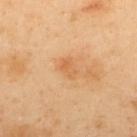The lesion was tiled from a total-body skin photograph and was not biopsied. Measured at roughly 2.5 mm in maximum diameter. The lesion is on the back. A 15 mm close-up extracted from a 3D total-body photography capture. A male subject aged around 40. This is a cross-polarized tile.